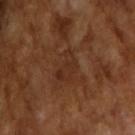No biopsy was performed on this lesion — it was imaged during a full skin examination and was not determined to be concerning. This image is a 15 mm lesion crop taken from a total-body photograph. The lesion's longest dimension is about 3.5 mm. Automated tile analysis of the lesion measured an outline eccentricity of about 0.8 (0 = round, 1 = elongated) and a shape-asymmetry score of about 0.45 (0 = symmetric). The software also gave a lesion–skin lightness drop of about 5 and a lesion-to-skin contrast of about 5 (normalized; higher = more distinct). The analysis additionally found a border-irregularity rating of about 4.5/10, internal color variation of about 2 on a 0–10 scale, and radial color variation of about 0.5. The software also gave a classifier nevus-likeness of about 0/100. A male subject about 65 years old.